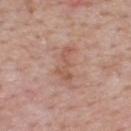<record>
  <biopsy_status>not biopsied; imaged during a skin examination</biopsy_status>
  <automated_metrics>
    <area_mm2_approx>5.0</area_mm2_approx>
    <eccentricity>0.9</eccentricity>
    <shape_asymmetry>0.5</shape_asymmetry>
    <cielab_L>55</cielab_L>
    <cielab_a>22</cielab_a>
    <cielab_b>28</cielab_b>
    <vs_skin_darker_L>8.0</vs_skin_darker_L>
    <vs_skin_contrast_norm>6.0</vs_skin_contrast_norm>
    <nevus_likeness_0_100>0</nevus_likeness_0_100>
    <lesion_detection_confidence_0_100>100</lesion_detection_confidence_0_100>
  </automated_metrics>
  <patient>
    <sex>male</sex>
    <age_approx>55</age_approx>
  </patient>
  <lesion_size>
    <long_diameter_mm_approx>4.0</long_diameter_mm_approx>
  </lesion_size>
  <image>
    <source>total-body photography crop</source>
    <field_of_view_mm>15</field_of_view_mm>
  </image>
  <lighting>white-light</lighting>
  <site>upper back</site>
</record>